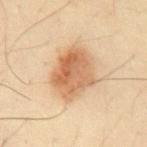Q: Was a biopsy performed?
A: catalogued during a skin exam; not biopsied
Q: Lesion location?
A: the chest
Q: What did automated image analysis measure?
A: a lesion area of about 20 mm²; an average lesion color of about L≈64 a*≈20 b*≈37 (CIELAB), about 13 CIELAB-L* units darker than the surrounding skin, and a lesion-to-skin contrast of about 8.5 (normalized; higher = more distinct)
Q: How was the tile lit?
A: cross-polarized
Q: What is the imaging modality?
A: total-body-photography crop, ~15 mm field of view
Q: Lesion size?
A: ~6 mm (longest diameter)
Q: What are the patient's age and sex?
A: male, aged around 50